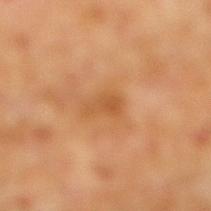Q: Was a biopsy performed?
A: total-body-photography surveillance lesion; no biopsy
Q: Illumination type?
A: cross-polarized
Q: Lesion location?
A: the right lower leg
Q: How large is the lesion?
A: about 3 mm
Q: Who is the patient?
A: male, aged approximately 60
Q: What did automated image analysis measure?
A: a mean CIELAB color near L≈51 a*≈23 b*≈40, about 7 CIELAB-L* units darker than the surrounding skin, and a normalized lesion–skin contrast near 6
Q: What kind of image is this?
A: total-body-photography crop, ~15 mm field of view